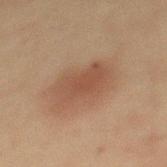Impression: Recorded during total-body skin imaging; not selected for excision or biopsy. Acquisition and patient details: The tile uses cross-polarized illumination. A male patient aged around 60. A region of skin cropped from a whole-body photographic capture, roughly 15 mm wide. The lesion is located on the mid back.